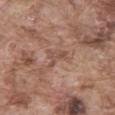<record>
  <biopsy_status>not biopsied; imaged during a skin examination</biopsy_status>
  <site>abdomen</site>
  <automated_metrics>
    <cielab_L>50</cielab_L>
    <cielab_a>19</cielab_a>
    <cielab_b>26</cielab_b>
    <vs_skin_darker_L>8.0</vs_skin_darker_L>
    <vs_skin_contrast_norm>6.0</vs_skin_contrast_norm>
  </automated_metrics>
  <lesion_size>
    <long_diameter_mm_approx>3.5</long_diameter_mm_approx>
  </lesion_size>
  <patient>
    <sex>male</sex>
    <age_approx>75</age_approx>
  </patient>
  <image>
    <source>total-body photography crop</source>
    <field_of_view_mm>15</field_of_view_mm>
  </image>
  <lighting>white-light</lighting>
</record>